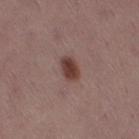Impression: Part of a total-body skin-imaging series; this lesion was reviewed on a skin check and was not flagged for biopsy. Context: The subject is a female aged 38 to 42. The lesion is on the right thigh. A lesion tile, about 15 mm wide, cut from a 3D total-body photograph.